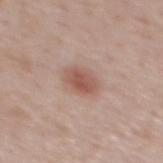Assessment: Recorded during total-body skin imaging; not selected for excision or biopsy. Context: Measured at roughly 3 mm in maximum diameter. Cropped from a whole-body photographic skin survey; the tile spans about 15 mm. The lesion-visualizer software estimated a lesion color around L≈54 a*≈22 b*≈26 in CIELAB, roughly 11 lightness units darker than nearby skin, and a normalized lesion–skin contrast near 7.5. The lesion is located on the mid back. The tile uses white-light illumination. A male patient, in their 40s.